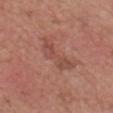The lesion was photographed on a routine skin check and not biopsied; there is no pathology result.